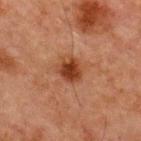<case>
  <biopsy_status>not biopsied; imaged during a skin examination</biopsy_status>
</case>